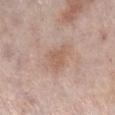Clinical impression: No biopsy was performed on this lesion — it was imaged during a full skin examination and was not determined to be concerning. Clinical summary: A male patient, in their 60s. The tile uses white-light illumination. An algorithmic analysis of the crop reported a lesion area of about 4.5 mm² and a symmetry-axis asymmetry near 0.4. The software also gave internal color variation of about 2 on a 0–10 scale and radial color variation of about 0.5. Approximately 2.5 mm at its widest. Cropped from a total-body skin-imaging series; the visible field is about 15 mm. From the right lower leg.